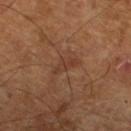Q: Was this lesion biopsied?
A: total-body-photography surveillance lesion; no biopsy
Q: Who is the patient?
A: male, in their mid-60s
Q: What is the imaging modality?
A: total-body-photography crop, ~15 mm field of view
Q: Lesion size?
A: about 3 mm
Q: What lighting was used for the tile?
A: cross-polarized
Q: What did automated image analysis measure?
A: a footprint of about 4 mm² and an outline eccentricity of about 0.85 (0 = round, 1 = elongated); a normalized border contrast of about 5
Q: Lesion location?
A: the left lower leg